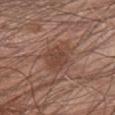The lesion was photographed on a routine skin check and not biopsied; there is no pathology result.